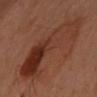| field | value |
|---|---|
| follow-up | imaged on a skin check; not biopsied |